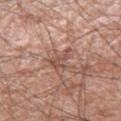{
  "biopsy_status": "not biopsied; imaged during a skin examination",
  "patient": {
    "sex": "male",
    "age_approx": 60
  },
  "automated_metrics": {
    "area_mm2_approx": 4.5,
    "eccentricity": 0.75,
    "shape_asymmetry": 0.55,
    "color_variation_0_10": 2.0,
    "peripheral_color_asymmetry": 0.5,
    "nevus_likeness_0_100": 0,
    "lesion_detection_confidence_0_100": 75
  },
  "lighting": "white-light",
  "image": {
    "source": "total-body photography crop",
    "field_of_view_mm": 15
  },
  "site": "right lower leg",
  "lesion_size": {
    "long_diameter_mm_approx": 3.0
  }
}